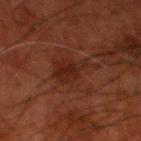The lesion was tiled from a total-body skin photograph and was not biopsied. This is a cross-polarized tile. Cropped from a total-body skin-imaging series; the visible field is about 15 mm. On the left lower leg. Automated image analysis of the tile measured a lesion area of about 8.5 mm², an outline eccentricity of about 0.8 (0 = round, 1 = elongated), and a symmetry-axis asymmetry near 0.45. And it measured a border-irregularity index near 5.5/10, internal color variation of about 2.5 on a 0–10 scale, and radial color variation of about 0.5. A male subject, aged around 80. The lesion's longest dimension is about 5 mm.